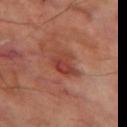Findings:
- biopsy status: no biopsy performed (imaged during a skin exam)
- TBP lesion metrics: a lesion area of about 7.5 mm², an eccentricity of roughly 0.7, and a symmetry-axis asymmetry near 0.35; a mean CIELAB color near L≈41 a*≈28 b*≈29, about 8 CIELAB-L* units darker than the surrounding skin, and a normalized lesion–skin contrast near 6.5; a border-irregularity index near 3.5/10, a color-variation rating of about 4.5/10, and peripheral color asymmetry of about 1.5; an automated nevus-likeness rating near 5 out of 100 and lesion-presence confidence of about 100/100
- illumination: cross-polarized
- location: the left thigh
- imaging modality: total-body-photography crop, ~15 mm field of view
- subject: male, in their 70s
- lesion size: ≈4 mm